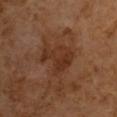Q: Is there a histopathology result?
A: total-body-photography surveillance lesion; no biopsy
Q: What did automated image analysis measure?
A: a lesion color around L≈31 a*≈20 b*≈29 in CIELAB, a lesion–skin lightness drop of about 7, and a lesion-to-skin contrast of about 6.5 (normalized; higher = more distinct); a nevus-likeness score of about 0/100 and a lesion-detection confidence of about 100/100
Q: Who is the patient?
A: male, aged 63 to 67
Q: Illumination type?
A: cross-polarized
Q: What is the imaging modality?
A: ~15 mm tile from a whole-body skin photo